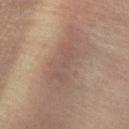workup — no biopsy performed (imaged during a skin exam)
subject — female, aged 43 to 47
acquisition — 15 mm crop, total-body photography
location — the right forearm
TBP lesion metrics — a footprint of about 9.5 mm², an eccentricity of roughly 0.9, and two-axis asymmetry of about 0.35; a lesion-to-skin contrast of about 5.5 (normalized; higher = more distinct); peripheral color asymmetry of about 0.5; an automated nevus-likeness rating near 0 out of 100 and a detector confidence of about 55 out of 100 that the crop contains a lesion